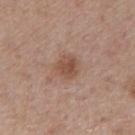size: about 2.5 mm | patient: male, about 55 years old | image: total-body-photography crop, ~15 mm field of view | lighting: white-light illumination | automated metrics: an area of roughly 6.5 mm², a shape eccentricity near 0.2, and a shape-asymmetry score of about 0.2 (0 = symmetric); a mean CIELAB color near L≈50 a*≈19 b*≈28, roughly 9 lightness units darker than nearby skin, and a normalized border contrast of about 7.